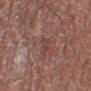Recorded during total-body skin imaging; not selected for excision or biopsy. The lesion is located on the right lower leg. A region of skin cropped from a whole-body photographic capture, roughly 15 mm wide. The patient is a male in their mid- to late 70s.